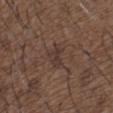follow-up: catalogued during a skin exam; not biopsied
subject: male, aged approximately 50
location: the upper back
acquisition: ~15 mm tile from a whole-body skin photo
lighting: white-light illumination
diameter: ~2.5 mm (longest diameter)